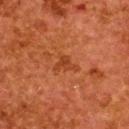Clinical impression:
Recorded during total-body skin imaging; not selected for excision or biopsy.